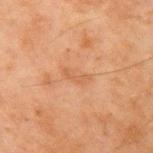| feature | finding |
|---|---|
| follow-up | no biopsy performed (imaged during a skin exam) |
| TBP lesion metrics | an area of roughly 3 mm², a shape eccentricity near 0.9, and a shape-asymmetry score of about 0.5 (0 = symmetric); an average lesion color of about L≈48 a*≈20 b*≈32 (CIELAB) and a normalized lesion–skin contrast near 4.5; a border-irregularity rating of about 5.5/10, a within-lesion color-variation index near 0/10, and a peripheral color-asymmetry measure near 0; a classifier nevus-likeness of about 0/100 and a detector confidence of about 100 out of 100 that the crop contains a lesion |
| diameter | ≈3 mm |
| subject | male, in their mid-40s |
| imaging modality | ~15 mm tile from a whole-body skin photo |
| tile lighting | cross-polarized illumination |
| anatomic site | the right upper arm |